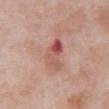{"biopsy_status": "not biopsied; imaged during a skin examination", "lighting": "white-light", "patient": {"sex": "male", "age_approx": 55}, "automated_metrics": {"area_mm2_approx": 8.0, "eccentricity": 0.9, "shape_asymmetry": 0.25, "border_irregularity_0_10": 3.0, "color_variation_0_10": 10.0, "nevus_likeness_0_100": 0, "lesion_detection_confidence_0_100": 100}, "lesion_size": {"long_diameter_mm_approx": 4.5}, "site": "front of the torso", "image": {"source": "total-body photography crop", "field_of_view_mm": 15}}